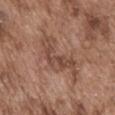This lesion was catalogued during total-body skin photography and was not selected for biopsy. The lesion is located on the front of the torso. This image is a 15 mm lesion crop taken from a total-body photograph. Approximately 7 mm at its widest. A male patient in their mid- to late 70s. The tile uses white-light illumination. The lesion-visualizer software estimated border irregularity of about 8.5 on a 0–10 scale, a color-variation rating of about 4/10, and a peripheral color-asymmetry measure near 1.5. It also reported a classifier nevus-likeness of about 0/100 and a lesion-detection confidence of about 80/100.